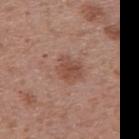follow-up = imaged on a skin check; not biopsied | tile lighting = white-light | anatomic site = the mid back | patient = male, roughly 70 years of age | acquisition = ~15 mm crop, total-body skin-cancer survey.